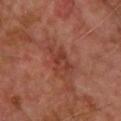Q: Was a biopsy performed?
A: imaged on a skin check; not biopsied
Q: What is the anatomic site?
A: the upper back
Q: Automated lesion metrics?
A: a footprint of about 7 mm², a shape eccentricity near 0.8, and a shape-asymmetry score of about 0.45 (0 = symmetric); a classifier nevus-likeness of about 10/100 and a detector confidence of about 100 out of 100 that the crop contains a lesion
Q: What lighting was used for the tile?
A: cross-polarized
Q: What are the patient's age and sex?
A: male, approximately 70 years of age
Q: Lesion size?
A: ~4 mm (longest diameter)
Q: How was this image acquired?
A: 15 mm crop, total-body photography